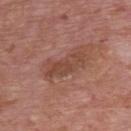No biopsy was performed on this lesion — it was imaged during a full skin examination and was not determined to be concerning.
This is a white-light tile.
A region of skin cropped from a whole-body photographic capture, roughly 15 mm wide.
Measured at roughly 5.5 mm in maximum diameter.
Located on the chest.
The total-body-photography lesion software estimated lesion-presence confidence of about 100/100.
The subject is a male approximately 75 years of age.